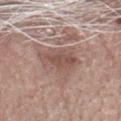Part of a total-body skin-imaging series; this lesion was reviewed on a skin check and was not flagged for biopsy. Located on the head or neck. The subject is a male roughly 70 years of age. The recorded lesion diameter is about 5 mm. A lesion tile, about 15 mm wide, cut from a 3D total-body photograph. The tile uses white-light illumination.